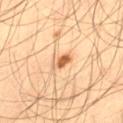The lesion was tiled from a total-body skin photograph and was not biopsied. This image is a 15 mm lesion crop taken from a total-body photograph. Located on the leg. Automated tile analysis of the lesion measured a footprint of about 3.5 mm², an eccentricity of roughly 0.8, and a symmetry-axis asymmetry near 0.3. The software also gave an average lesion color of about L≈61 a*≈22 b*≈39 (CIELAB) and a normalized lesion–skin contrast near 9. The analysis additionally found border irregularity of about 2.5 on a 0–10 scale, a within-lesion color-variation index near 5/10, and peripheral color asymmetry of about 1.5. The software also gave a detector confidence of about 100 out of 100 that the crop contains a lesion. A male patient, aged around 45.